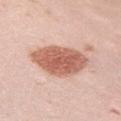– workup · imaged on a skin check; not biopsied
– lighting · white-light illumination
– patient · female, in their 30s
– location · the right upper arm
– automated metrics · a lesion area of about 21 mm² and a shape eccentricity near 0.8; peripheral color asymmetry of about 1
– lesion size · ≈7 mm
– image · ~15 mm crop, total-body skin-cancer survey